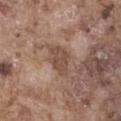notes — catalogued during a skin exam; not biopsied | acquisition — 15 mm crop, total-body photography | patient — male, in their mid- to late 70s | illumination — white-light | site — the abdomen | diameter — ≈3.5 mm.